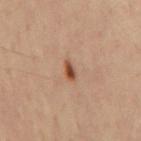  biopsy_status: not biopsied; imaged during a skin examination
  site: mid back
  patient:
    sex: male
    age_approx: 65
  lesion_size:
    long_diameter_mm_approx: 2.5
  image:
    source: total-body photography crop
    field_of_view_mm: 15